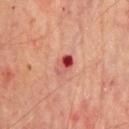Case summary:
• notes — catalogued during a skin exam; not biopsied
• imaging modality — ~15 mm tile from a whole-body skin photo
• patient — male, aged approximately 65
• diameter — ~3 mm (longest diameter)
• body site — the chest
• illumination — cross-polarized illumination